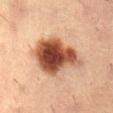Background: Measured at roughly 7 mm in maximum diameter. Automated image analysis of the tile measured an area of roughly 24 mm², a shape eccentricity near 0.75, and a shape-asymmetry score of about 0.25 (0 = symmetric). The analysis additionally found an average lesion color of about L≈43 a*≈22 b*≈30 (CIELAB), about 19 CIELAB-L* units darker than the surrounding skin, and a normalized lesion–skin contrast near 13.5. And it measured a border-irregularity index near 3/10, internal color variation of about 8.5 on a 0–10 scale, and peripheral color asymmetry of about 2.5. And it measured a nevus-likeness score of about 100/100 and a detector confidence of about 100 out of 100 that the crop contains a lesion. A male patient, in their mid-50s. The tile uses cross-polarized illumination. A 15 mm close-up tile from a total-body photography series done for melanoma screening. The lesion is located on the back.